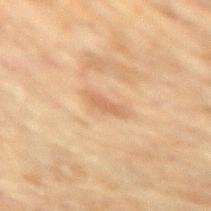• biopsy status — total-body-photography surveillance lesion; no biopsy
• image — total-body-photography crop, ~15 mm field of view
• body site — the arm
• patient — female, in their 80s
• lesion diameter — ≈2.5 mm